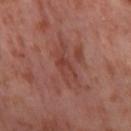Impression: Part of a total-body skin-imaging series; this lesion was reviewed on a skin check and was not flagged for biopsy. Background: On the leg. A roughly 15 mm field-of-view crop from a total-body skin photograph. A female patient roughly 55 years of age.